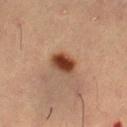| key | value |
|---|---|
| image-analysis metrics | a border-irregularity index near 2/10, a within-lesion color-variation index near 4.5/10, and radial color variation of about 1 |
| image | ~15 mm tile from a whole-body skin photo |
| site | the leg |
| patient | male, in their mid-50s |
| size | ~3 mm (longest diameter) |